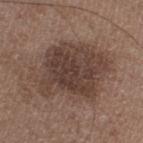Assessment:
Imaged during a routine full-body skin examination; the lesion was not biopsied and no histopathology is available.
Context:
Located on the left lower leg. This is a white-light tile. This image is a 15 mm lesion crop taken from a total-body photograph. A male subject in their 50s.